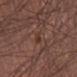Notes:
• notes: no biopsy performed (imaged during a skin exam)
• acquisition: ~15 mm crop, total-body skin-cancer survey
• diameter: ~3 mm (longest diameter)
• subject: male, approximately 55 years of age
• body site: the right lower leg